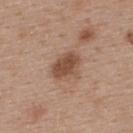Q: What is the imaging modality?
A: ~15 mm tile from a whole-body skin photo
Q: Automated lesion metrics?
A: an eccentricity of roughly 0.65 and a symmetry-axis asymmetry near 0.2; a mean CIELAB color near L≈49 a*≈19 b*≈29, about 11 CIELAB-L* units darker than the surrounding skin, and a lesion-to-skin contrast of about 8 (normalized; higher = more distinct); a color-variation rating of about 2.5/10 and radial color variation of about 1
Q: Where on the body is the lesion?
A: the upper back
Q: What lighting was used for the tile?
A: white-light
Q: Who is the patient?
A: female, aged around 40